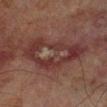Clinical impression:
Recorded during total-body skin imaging; not selected for excision or biopsy.
Background:
From the right lower leg. A roughly 15 mm field-of-view crop from a total-body skin photograph. A male subject, approximately 70 years of age.